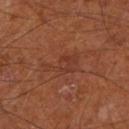Clinical impression:
No biopsy was performed on this lesion — it was imaged during a full skin examination and was not determined to be concerning.
Image and clinical context:
The lesion is on the left lower leg. The total-body-photography lesion software estimated border irregularity of about 6.5 on a 0–10 scale, internal color variation of about 2.5 on a 0–10 scale, and radial color variation of about 0.5. It also reported a nevus-likeness score of about 0/100 and a lesion-detection confidence of about 100/100. The subject is a male in their mid-60s. Cropped from a whole-body photographic skin survey; the tile spans about 15 mm. The tile uses cross-polarized illumination.